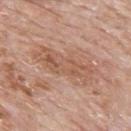This image is a 15 mm lesion crop taken from a total-body photograph.
On the back.
A male patient aged around 80.
Approximately 7.5 mm at its widest.
Captured under white-light illumination.